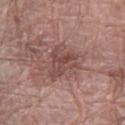Q: Was a biopsy performed?
A: imaged on a skin check; not biopsied
Q: How large is the lesion?
A: ~4.5 mm (longest diameter)
Q: What kind of image is this?
A: ~15 mm crop, total-body skin-cancer survey
Q: Where on the body is the lesion?
A: the left forearm
Q: How was the tile lit?
A: white-light illumination
Q: Who is the patient?
A: male, aged approximately 65